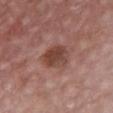No biopsy was performed on this lesion — it was imaged during a full skin examination and was not determined to be concerning. A male subject in their mid- to late 80s or older. The recorded lesion diameter is about 3.5 mm. A close-up tile cropped from a whole-body skin photograph, about 15 mm across. This is a white-light tile. Located on the chest. An algorithmic analysis of the crop reported an eccentricity of roughly 0.55 and a symmetry-axis asymmetry near 0.2. And it measured a border-irregularity index near 1.5/10. The software also gave a nevus-likeness score of about 5/100 and a lesion-detection confidence of about 100/100.